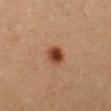Q: Was a biopsy performed?
A: catalogued during a skin exam; not biopsied
Q: What kind of image is this?
A: total-body-photography crop, ~15 mm field of view
Q: What lighting was used for the tile?
A: cross-polarized illumination
Q: Lesion location?
A: the left lower leg
Q: What did automated image analysis measure?
A: a footprint of about 5.5 mm², a shape eccentricity near 0.45, and a symmetry-axis asymmetry near 0.15
Q: Who is the patient?
A: female, in their mid- to late 20s